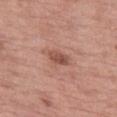Assessment: Imaged during a routine full-body skin examination; the lesion was not biopsied and no histopathology is available. Clinical summary: About 3 mm across. The patient is a female aged approximately 70. A close-up tile cropped from a whole-body skin photograph, about 15 mm across. The lesion is on the left thigh. The lesion-visualizer software estimated an area of roughly 4 mm² and an outline eccentricity of about 0.85 (0 = round, 1 = elongated). And it measured internal color variation of about 3 on a 0–10 scale and a peripheral color-asymmetry measure near 1. Captured under white-light illumination.